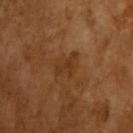The lesion was tiled from a total-body skin photograph and was not biopsied. This is a cross-polarized tile. The subject is a male in their mid- to late 60s. Longest diameter approximately 3.5 mm. A region of skin cropped from a whole-body photographic capture, roughly 15 mm wide.